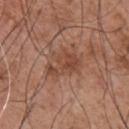No biopsy was performed on this lesion — it was imaged during a full skin examination and was not determined to be concerning. This is a white-light tile. Approximately 4.5 mm at its widest. This image is a 15 mm lesion crop taken from a total-body photograph. The lesion is located on the chest. A male patient aged 53 to 57. Automated image analysis of the tile measured a footprint of about 8 mm², a shape eccentricity near 0.8, and two-axis asymmetry of about 0.4. The software also gave about 8 CIELAB-L* units darker than the surrounding skin and a normalized lesion–skin contrast near 6.5. And it measured a border-irregularity rating of about 5.5/10, a color-variation rating of about 3.5/10, and radial color variation of about 1.5.